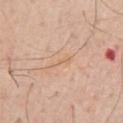Part of a total-body skin-imaging series; this lesion was reviewed on a skin check and was not flagged for biopsy.
A male patient aged around 70.
Captured under white-light illumination.
A 15 mm close-up tile from a total-body photography series done for melanoma screening.
Measured at roughly 3 mm in maximum diameter.
Located on the chest.